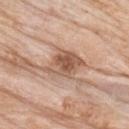Notes:
- subject: male, roughly 85 years of age or older
- location: the back
- tile lighting: white-light illumination
- acquisition: ~15 mm tile from a whole-body skin photo
- size: ≈5.5 mm
- TBP lesion metrics: an area of roughly 12 mm²; a mean CIELAB color near L≈57 a*≈20 b*≈30, roughly 12 lightness units darker than nearby skin, and a lesion-to-skin contrast of about 8 (normalized; higher = more distinct)